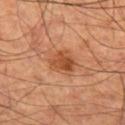Assessment:
Recorded during total-body skin imaging; not selected for excision or biopsy.
Context:
A male subject, roughly 70 years of age. Imaged with cross-polarized lighting. A 15 mm close-up extracted from a 3D total-body photography capture. Longest diameter approximately 3.5 mm. The lesion-visualizer software estimated an automated nevus-likeness rating near 65 out of 100. The lesion is located on the left thigh.